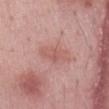<record>
  <biopsy_status>not biopsied; imaged during a skin examination</biopsy_status>
  <lighting>white-light</lighting>
  <lesion_size>
    <long_diameter_mm_approx>4.5</long_diameter_mm_approx>
  </lesion_size>
  <patient>
    <sex>male</sex>
    <age_approx>65</age_approx>
  </patient>
  <site>mid back</site>
  <image>
    <source>total-body photography crop</source>
    <field_of_view_mm>15</field_of_view_mm>
  </image>
  <automated_metrics>
    <area_mm2_approx>7.5</area_mm2_approx>
    <eccentricity>0.85</eccentricity>
    <shape_asymmetry>0.3</shape_asymmetry>
    <cielab_L>58</cielab_L>
    <cielab_a>24</cielab_a>
    <cielab_b>25</cielab_b>
    <vs_skin_darker_L>7.0</vs_skin_darker_L>
    <nevus_likeness_0_100>0</nevus_likeness_0_100>
    <lesion_detection_confidence_0_100>100</lesion_detection_confidence_0_100>
  </automated_metrics>
</record>